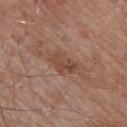Impression: Imaged during a routine full-body skin examination; the lesion was not biopsied and no histopathology is available. Acquisition and patient details: The lesion is located on the upper back. Imaged with white-light lighting. The recorded lesion diameter is about 3.5 mm. A close-up tile cropped from a whole-body skin photograph, about 15 mm across. A male patient in their mid-50s.